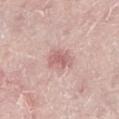Clinical impression:
Imaged during a routine full-body skin examination; the lesion was not biopsied and no histopathology is available.
Image and clinical context:
The patient is a female roughly 65 years of age. Cropped from a whole-body photographic skin survey; the tile spans about 15 mm. The lesion-visualizer software estimated a border-irregularity index near 2.5/10 and internal color variation of about 2.5 on a 0–10 scale. Measured at roughly 3.5 mm in maximum diameter. The tile uses white-light illumination. The lesion is located on the left lower leg.